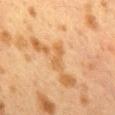follow-up = imaged on a skin check; not biopsied
site = the mid back
patient = female, aged approximately 40
image source = ~15 mm crop, total-body skin-cancer survey
tile lighting = cross-polarized illumination
lesion diameter = about 3.5 mm